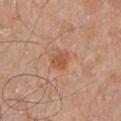notes: imaged on a skin check; not biopsied
image: 15 mm crop, total-body photography
subject: male, roughly 80 years of age
body site: the left upper arm
tile lighting: white-light
lesion diameter: ~2.5 mm (longest diameter)